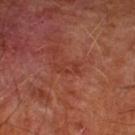Findings:
* workup: total-body-photography surveillance lesion; no biopsy
* image source: 15 mm crop, total-body photography
* lesion diameter: ~3 mm (longest diameter)
* site: the left lower leg
* automated metrics: a mean CIELAB color near L≈36 a*≈27 b*≈28, a lesion–skin lightness drop of about 5, and a lesion-to-skin contrast of about 5 (normalized; higher = more distinct); a nevus-likeness score of about 0/100 and lesion-presence confidence of about 100/100
* subject: male, aged around 65